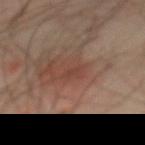Captured under cross-polarized illumination.
A roughly 15 mm field-of-view crop from a total-body skin photograph.
Measured at roughly 1.5 mm in maximum diameter.
A male subject in their mid-60s.
Automated tile analysis of the lesion measured a lesion area of about 1 mm², an outline eccentricity of about 0.85 (0 = round, 1 = elongated), and two-axis asymmetry of about 0.5. The software also gave a mean CIELAB color near L≈39 a*≈21 b*≈25, about 5 CIELAB-L* units darker than the surrounding skin, and a normalized lesion–skin contrast near 4.5. And it measured a border-irregularity rating of about 4/10, a color-variation rating of about 0/10, and radial color variation of about 0.
Located on the abdomen.